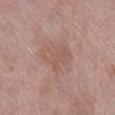Part of a total-body skin-imaging series; this lesion was reviewed on a skin check and was not flagged for biopsy.
This is a white-light tile.
Automated tile analysis of the lesion measured a shape eccentricity near 0.75 and a symmetry-axis asymmetry near 0.4. The software also gave border irregularity of about 6 on a 0–10 scale, internal color variation of about 1.5 on a 0–10 scale, and radial color variation of about 0.5. And it measured a classifier nevus-likeness of about 0/100 and a lesion-detection confidence of about 100/100.
A 15 mm crop from a total-body photograph taken for skin-cancer surveillance.
A female subject, aged approximately 70.
Located on the right lower leg.
About 4 mm across.